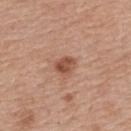workup: no biopsy performed (imaged during a skin exam) | subject: female, roughly 60 years of age | size: ≈2.5 mm | body site: the back | image source: ~15 mm tile from a whole-body skin photo.